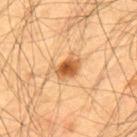Assessment:
Recorded during total-body skin imaging; not selected for excision or biopsy.
Image and clinical context:
The lesion is on the upper back. A close-up tile cropped from a whole-body skin photograph, about 15 mm across. Captured under cross-polarized illumination. A male patient, aged approximately 55.